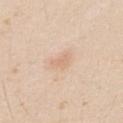Assessment: Imaged during a routine full-body skin examination; the lesion was not biopsied and no histopathology is available. Background: The lesion is on the upper back. The total-body-photography lesion software estimated a border-irregularity rating of about 3.5/10, internal color variation of about 1 on a 0–10 scale, and peripheral color asymmetry of about 0.5. The subject is a male roughly 25 years of age. A 15 mm close-up extracted from a 3D total-body photography capture.